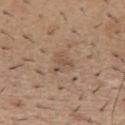biopsy status: imaged on a skin check; not biopsied | TBP lesion metrics: an area of roughly 4 mm², an eccentricity of roughly 0.55, and a shape-asymmetry score of about 0.55 (0 = symmetric); border irregularity of about 5 on a 0–10 scale, a color-variation rating of about 1/10, and peripheral color asymmetry of about 0.5 | patient: male, about 40 years old | diameter: ~2.5 mm (longest diameter) | image source: total-body-photography crop, ~15 mm field of view | body site: the mid back | illumination: white-light.